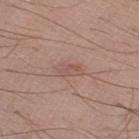Part of a total-body skin-imaging series; this lesion was reviewed on a skin check and was not flagged for biopsy. The lesion is located on the leg. Automated tile analysis of the lesion measured a mean CIELAB color near L≈53 a*≈20 b*≈24, roughly 7 lightness units darker than nearby skin, and a normalized border contrast of about 5. The lesion's longest dimension is about 2.5 mm. A male subject, aged around 20. This is a white-light tile. A lesion tile, about 15 mm wide, cut from a 3D total-body photograph.